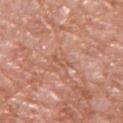Q: Was a biopsy performed?
A: catalogued during a skin exam; not biopsied
Q: What is the anatomic site?
A: the upper back
Q: How large is the lesion?
A: about 2.5 mm
Q: What is the imaging modality?
A: ~15 mm crop, total-body skin-cancer survey
Q: What are the patient's age and sex?
A: male, about 55 years old
Q: What lighting was used for the tile?
A: white-light illumination